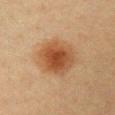biopsy status = catalogued during a skin exam; not biopsied | subject = female, aged approximately 40 | TBP lesion metrics = a footprint of about 19 mm²; an average lesion color of about L≈44 a*≈20 b*≈33 (CIELAB), roughly 10 lightness units darker than nearby skin, and a normalized border contrast of about 8.5; a nevus-likeness score of about 100/100 and a detector confidence of about 100 out of 100 that the crop contains a lesion | tile lighting = cross-polarized | body site = the chest | lesion diameter = ≈5 mm | imaging modality = 15 mm crop, total-body photography.